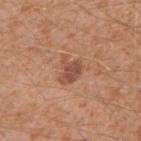notes: no biopsy performed (imaged during a skin exam) | image: 15 mm crop, total-body photography | body site: the right upper arm | lighting: white-light illumination | automated metrics: a nevus-likeness score of about 25/100 and lesion-presence confidence of about 100/100 | patient: male, aged 28 to 32.